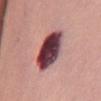– notes — catalogued during a skin exam; not biopsied
– acquisition — total-body-photography crop, ~15 mm field of view
– patient — female, in their mid-60s
– illumination — white-light
– image-analysis metrics — a footprint of about 17 mm² and a shape-asymmetry score of about 0.1 (0 = symmetric)
– location — the chest
– diameter — ~6.5 mm (longest diameter)